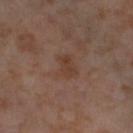follow-up: no biopsy performed (imaged during a skin exam) | image source: ~15 mm crop, total-body skin-cancer survey | patient: female, aged approximately 55 | size: about 3.5 mm | site: the left thigh | illumination: cross-polarized.